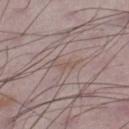notes: total-body-photography surveillance lesion; no biopsy
lighting: white-light
automated metrics: a lesion area of about 3 mm², an eccentricity of roughly 0.9, and a symmetry-axis asymmetry near 0.55; an average lesion color of about L≈53 a*≈15 b*≈21 (CIELAB), about 5 CIELAB-L* units darker than the surrounding skin, and a lesion-to-skin contrast of about 4.5 (normalized; higher = more distinct); a border-irregularity rating of about 6/10, a within-lesion color-variation index near 0/10, and peripheral color asymmetry of about 0
imaging modality: total-body-photography crop, ~15 mm field of view
site: the right lower leg
subject: male, aged 48–52